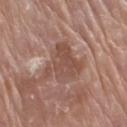* location — the right upper arm
* size — about 4.5 mm
* patient — male, approximately 80 years of age
* tile lighting — white-light
* imaging modality — ~15 mm crop, total-body skin-cancer survey
* automated lesion analysis — a border-irregularity index near 6/10, a within-lesion color-variation index near 2.5/10, and peripheral color asymmetry of about 1